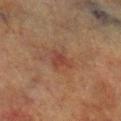notes — imaged on a skin check; not biopsied | image source — total-body-photography crop, ~15 mm field of view | illumination — cross-polarized illumination | TBP lesion metrics — a lesion area of about 4 mm² and a symmetry-axis asymmetry near 0.5; a mean CIELAB color near L≈34 a*≈21 b*≈24, about 6 CIELAB-L* units darker than the surrounding skin, and a lesion-to-skin contrast of about 6 (normalized; higher = more distinct); a border-irregularity index near 5/10 and a color-variation rating of about 1.5/10; a nevus-likeness score of about 0/100 and a lesion-detection confidence of about 100/100 | patient — male, about 70 years old | site — the left lower leg | lesion diameter — about 2.5 mm.